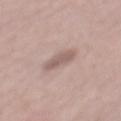On the back. About 4 mm across. The subject is a male about 60 years old. This image is a 15 mm lesion crop taken from a total-body photograph. Automated tile analysis of the lesion measured a lesion area of about 5.5 mm² and an eccentricity of roughly 0.9. It also reported a mean CIELAB color near L≈58 a*≈16 b*≈21, roughly 10 lightness units darker than nearby skin, and a lesion-to-skin contrast of about 6.5 (normalized; higher = more distinct). It also reported a nevus-likeness score of about 0/100 and lesion-presence confidence of about 95/100.